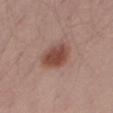Captured during whole-body skin photography for melanoma surveillance; the lesion was not biopsied. The patient is a male roughly 55 years of age. The lesion is located on the right thigh. The tile uses white-light illumination. Measured at roughly 4.5 mm in maximum diameter. Automated image analysis of the tile measured a lesion color around L≈47 a*≈22 b*≈26 in CIELAB, about 12 CIELAB-L* units darker than the surrounding skin, and a lesion-to-skin contrast of about 9 (normalized; higher = more distinct). It also reported an automated nevus-likeness rating near 100 out of 100 and lesion-presence confidence of about 100/100. Cropped from a total-body skin-imaging series; the visible field is about 15 mm.